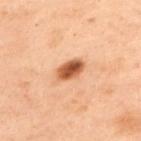Q: Is there a histopathology result?
A: catalogued during a skin exam; not biopsied
Q: Who is the patient?
A: female, aged 53 to 57
Q: Where on the body is the lesion?
A: the upper back
Q: What kind of image is this?
A: 15 mm crop, total-body photography